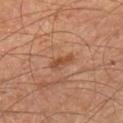workup=total-body-photography surveillance lesion; no biopsy
tile lighting=cross-polarized illumination
diameter=≈3 mm
patient=male, aged around 65
site=the upper back
image=total-body-photography crop, ~15 mm field of view